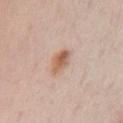Assessment:
Part of a total-body skin-imaging series; this lesion was reviewed on a skin check and was not flagged for biopsy.